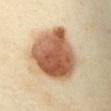{
  "biopsy_status": "not biopsied; imaged during a skin examination",
  "patient": {
    "sex": "female",
    "age_approx": 55
  },
  "site": "front of the torso",
  "lighting": "cross-polarized",
  "automated_metrics": {
    "eccentricity": 0.55,
    "shape_asymmetry": 0.15,
    "border_irregularity_0_10": 1.5,
    "color_variation_0_10": 8.0,
    "peripheral_color_asymmetry": 3.0,
    "nevus_likeness_0_100": 100
  },
  "image": {
    "source": "total-body photography crop",
    "field_of_view_mm": 15
  }
}